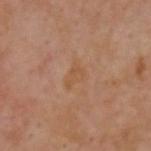A close-up tile cropped from a whole-body skin photograph, about 15 mm across. A male subject, aged 53 to 57. The tile uses cross-polarized illumination. The lesion is located on the upper back. About 3 mm across.